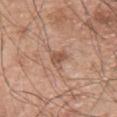Impression:
Imaged during a routine full-body skin examination; the lesion was not biopsied and no histopathology is available.
Context:
Automated tile analysis of the lesion measured an eccentricity of roughly 0.7 and a shape-asymmetry score of about 0.45 (0 = symmetric). And it measured border irregularity of about 4.5 on a 0–10 scale, a within-lesion color-variation index near 2/10, and a peripheral color-asymmetry measure near 0.5. A male patient, aged 63–67. Approximately 2.5 mm at its widest. Imaged with white-light lighting. From the chest. A roughly 15 mm field-of-view crop from a total-body skin photograph.